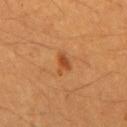Q: Lesion location?
A: the mid back
Q: Who is the patient?
A: male, aged around 60
Q: What did automated image analysis measure?
A: an area of roughly 4 mm² and a shape eccentricity near 0.85; a lesion color around L≈45 a*≈25 b*≈39 in CIELAB and a lesion-to-skin contrast of about 7 (normalized; higher = more distinct); a border-irregularity rating of about 4.5/10
Q: How was this image acquired?
A: ~15 mm tile from a whole-body skin photo